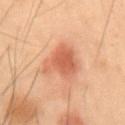follow-up = catalogued during a skin exam; not biopsied | tile lighting = cross-polarized illumination | location = the abdomen | subject = male, aged around 55 | image source = ~15 mm tile from a whole-body skin photo.